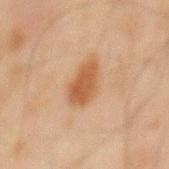follow-up = imaged on a skin check; not biopsied | patient = male, approximately 45 years of age | image = 15 mm crop, total-body photography | lesion diameter = ~4.5 mm (longest diameter) | tile lighting = cross-polarized | body site = the mid back.